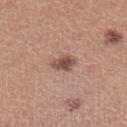| field | value |
|---|---|
| workup | total-body-photography surveillance lesion; no biopsy |
| lesion size | about 3 mm |
| image | 15 mm crop, total-body photography |
| site | the left lower leg |
| subject | female, about 40 years old |
| lighting | white-light |
| automated lesion analysis | a lesion area of about 4.5 mm²; a color-variation rating of about 3.5/10 and radial color variation of about 1 |